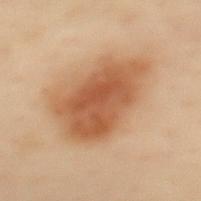Q: Was this lesion biopsied?
A: imaged on a skin check; not biopsied
Q: Illumination type?
A: cross-polarized illumination
Q: How large is the lesion?
A: ≈7.5 mm
Q: Where on the body is the lesion?
A: the upper back
Q: How was this image acquired?
A: ~15 mm crop, total-body skin-cancer survey
Q: Patient demographics?
A: female, aged approximately 60